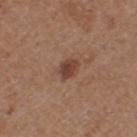The lesion was photographed on a routine skin check and not biopsied; there is no pathology result. From the leg. The lesion-visualizer software estimated an area of roughly 4 mm² and an outline eccentricity of about 0.7 (0 = round, 1 = elongated). And it measured border irregularity of about 3 on a 0–10 scale and a within-lesion color-variation index near 2.5/10. Approximately 2.5 mm at its widest. A 15 mm close-up extracted from a 3D total-body photography capture. This is a white-light tile. The subject is a female roughly 40 years of age.